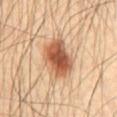  biopsy_status: not biopsied; imaged during a skin examination
  site: abdomen
  lighting: cross-polarized
  patient:
    sex: male
    age_approx: 60
  image:
    source: total-body photography crop
    field_of_view_mm: 15